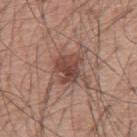<lesion>
<biopsy_status>not biopsied; imaged during a skin examination</biopsy_status>
<image>
  <source>total-body photography crop</source>
  <field_of_view_mm>15</field_of_view_mm>
</image>
<patient>
  <sex>male</sex>
  <age_approx>55</age_approx>
</patient>
<site>back</site>
</lesion>